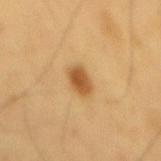The lesion was photographed on a routine skin check and not biopsied; there is no pathology result. A region of skin cropped from a whole-body photographic capture, roughly 15 mm wide. The lesion's longest dimension is about 3.5 mm. Located on the mid back. The patient is a male in their 60s. This is a cross-polarized tile.